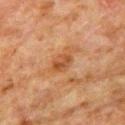Findings:
• workup: no biopsy performed (imaged during a skin exam)
• TBP lesion metrics: an area of roughly 4 mm² and an eccentricity of roughly 0.7; internal color variation of about 4 on a 0–10 scale and peripheral color asymmetry of about 1.5
• patient: male, in their 80s
• location: the left upper arm
• illumination: cross-polarized illumination
• image source: 15 mm crop, total-body photography
• lesion diameter: ≈2.5 mm